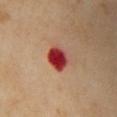{
  "biopsy_status": "not biopsied; imaged during a skin examination",
  "lighting": "cross-polarized",
  "image": {
    "source": "total-body photography crop",
    "field_of_view_mm": 15
  },
  "site": "chest",
  "patient": {
    "sex": "female",
    "age_approx": 55
  },
  "lesion_size": {
    "long_diameter_mm_approx": 3.0
  }
}